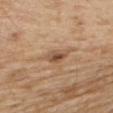Case summary:
– notes · catalogued during a skin exam; not biopsied
– TBP lesion metrics · an average lesion color of about L≈52 a*≈18 b*≈32 (CIELAB), a lesion–skin lightness drop of about 10, and a lesion-to-skin contrast of about 7 (normalized; higher = more distinct)
– image source · total-body-photography crop, ~15 mm field of view
– site · the upper back
– lighting · white-light illumination
– patient · male, aged 68–72